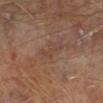Part of a total-body skin-imaging series; this lesion was reviewed on a skin check and was not flagged for biopsy.
The patient is a male aged 63–67.
The tile uses cross-polarized illumination.
Cropped from a whole-body photographic skin survey; the tile spans about 15 mm.
The lesion is on the leg.
Longest diameter approximately 4 mm.
The lesion-visualizer software estimated a lesion area of about 3.5 mm², a shape eccentricity near 0.95, and a symmetry-axis asymmetry near 0.65. The analysis additionally found an average lesion color of about L≈41 a*≈19 b*≈24 (CIELAB) and a normalized lesion–skin contrast near 4.5. It also reported a color-variation rating of about 0/10 and peripheral color asymmetry of about 0.